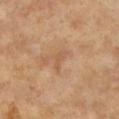Imaged during a routine full-body skin examination; the lesion was not biopsied and no histopathology is available. A roughly 15 mm field-of-view crop from a total-body skin photograph. A female patient, about 75 years old. This is a cross-polarized tile. The lesion is located on the upper back. The lesion's longest dimension is about 2.5 mm. The lesion-visualizer software estimated a lesion area of about 2.5 mm². The software also gave a border-irregularity rating of about 4.5/10 and radial color variation of about 0. And it measured an automated nevus-likeness rating near 0 out of 100 and a lesion-detection confidence of about 100/100.